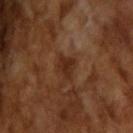Impression:
This lesion was catalogued during total-body skin photography and was not selected for biopsy.
Background:
Cropped from a total-body skin-imaging series; the visible field is about 15 mm. The recorded lesion diameter is about 2.5 mm. This is a cross-polarized tile. The patient is a male in their mid- to late 60s. An algorithmic analysis of the crop reported an area of roughly 4 mm² and a symmetry-axis asymmetry near 0.3.